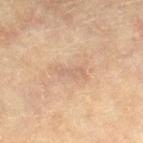No biopsy was performed on this lesion — it was imaged during a full skin examination and was not determined to be concerning. Located on the right lower leg. Measured at roughly 3.5 mm in maximum diameter. A roughly 15 mm field-of-view crop from a total-body skin photograph. The subject is a female aged around 80.